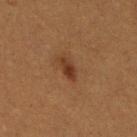Clinical impression: The lesion was tiled from a total-body skin photograph and was not biopsied. Clinical summary: This is a cross-polarized tile. A female patient, approximately 30 years of age. A close-up tile cropped from a whole-body skin photograph, about 15 mm across. From the left leg. The lesion-visualizer software estimated an average lesion color of about L≈33 a*≈21 b*≈30 (CIELAB), a lesion–skin lightness drop of about 9, and a normalized lesion–skin contrast near 8.5.